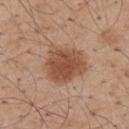No biopsy was performed on this lesion — it was imaged during a full skin examination and was not determined to be concerning. Located on the upper back. The total-body-photography lesion software estimated an area of roughly 17 mm², a shape eccentricity near 0.5, and a shape-asymmetry score of about 0.25 (0 = symmetric). The analysis additionally found a border-irregularity index near 2.5/10 and radial color variation of about 1. The software also gave an automated nevus-likeness rating near 90 out of 100 and a lesion-detection confidence of about 100/100. The recorded lesion diameter is about 5 mm. This image is a 15 mm lesion crop taken from a total-body photograph. Imaged with white-light lighting. A male subject, approximately 55 years of age.